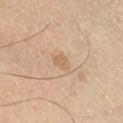Recorded during total-body skin imaging; not selected for excision or biopsy.
From the right lower leg.
A male patient aged 58–62.
A 15 mm close-up extracted from a 3D total-body photography capture.
The lesion's longest dimension is about 2.5 mm.
This is a cross-polarized tile.